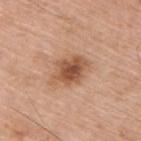{
  "biopsy_status": "not biopsied; imaged during a skin examination",
  "site": "upper back",
  "image": {
    "source": "total-body photography crop",
    "field_of_view_mm": 15
  },
  "patient": {
    "sex": "male",
    "age_approx": 75
  }
}